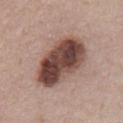The total-body-photography lesion software estimated a lesion area of about 26 mm² and an eccentricity of roughly 0.8. And it measured an average lesion color of about L≈44 a*≈20 b*≈23 (CIELAB).
A male patient aged 53 to 57.
About 7.5 mm across.
On the abdomen.
A 15 mm close-up extracted from a 3D total-body photography capture.
Imaged with white-light lighting.